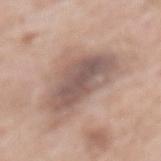Findings:
– follow-up — imaged on a skin check; not biopsied
– subject — female, about 75 years old
– acquisition — total-body-photography crop, ~15 mm field of view
– lesion diameter — ~7 mm (longest diameter)
– lighting — white-light illumination
– image-analysis metrics — a footprint of about 22 mm², an outline eccentricity of about 0.85 (0 = round, 1 = elongated), and two-axis asymmetry of about 0.25; internal color variation of about 5 on a 0–10 scale and peripheral color asymmetry of about 1.5; lesion-presence confidence of about 95/100
– anatomic site — the mid back